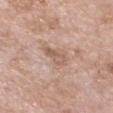This lesion was catalogued during total-body skin photography and was not selected for biopsy. From the chest. An algorithmic analysis of the crop reported an eccentricity of roughly 0.9 and two-axis asymmetry of about 0.3. The analysis additionally found a mean CIELAB color near L≈59 a*≈18 b*≈28, roughly 9 lightness units darker than nearby skin, and a normalized border contrast of about 6. Longest diameter approximately 4 mm. A female subject, in their mid-70s. Cropped from a whole-body photographic skin survey; the tile spans about 15 mm.